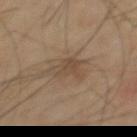Imaged during a routine full-body skin examination; the lesion was not biopsied and no histopathology is available.
A 15 mm crop from a total-body photograph taken for skin-cancer surveillance.
The subject is a male about 65 years old.
The lesion is located on the chest.